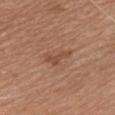{"biopsy_status": "not biopsied; imaged during a skin examination", "patient": {"sex": "female", "age_approx": 60}, "image": {"source": "total-body photography crop", "field_of_view_mm": 15}, "site": "chest", "lesion_size": {"long_diameter_mm_approx": 3.0}, "automated_metrics": {"area_mm2_approx": 3.5, "eccentricity": 0.9, "border_irregularity_0_10": 5.5, "peripheral_color_asymmetry": 0.0, "nevus_likeness_0_100": 5, "lesion_detection_confidence_0_100": 100}, "lighting": "white-light"}